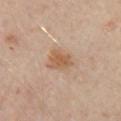The lesion was photographed on a routine skin check and not biopsied; there is no pathology result. From the front of the torso. This image is a 15 mm lesion crop taken from a total-body photograph. A subject aged 53–57. This is a cross-polarized tile.